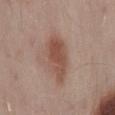biopsy status: catalogued during a skin exam; not biopsied | patient: male, aged approximately 55 | image source: ~15 mm crop, total-body skin-cancer survey | lesion diameter: ~6 mm (longest diameter) | lighting: white-light | image-analysis metrics: a border-irregularity index near 2.5/10, a within-lesion color-variation index near 3.5/10, and radial color variation of about 1 | body site: the back.